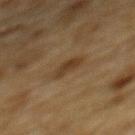biopsy status: no biopsy performed (imaged during a skin exam) | diameter: about 2.5 mm | imaging modality: ~15 mm crop, total-body skin-cancer survey | patient: male, in their mid-80s or older | anatomic site: the mid back | illumination: cross-polarized | automated lesion analysis: a border-irregularity index near 2/10, a within-lesion color-variation index near 2/10, and peripheral color asymmetry of about 1; a classifier nevus-likeness of about 50/100 and a detector confidence of about 100 out of 100 that the crop contains a lesion.